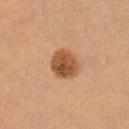location=the leg
subject=female, aged 28–32
image=~15 mm crop, total-body skin-cancer survey